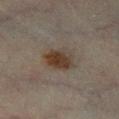biopsy status=imaged on a skin check; not biopsied.